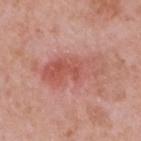Clinical impression:
Recorded during total-body skin imaging; not selected for excision or biopsy.
Clinical summary:
Located on the upper back. A male patient, in their 50s. Cropped from a whole-body photographic skin survey; the tile spans about 15 mm.